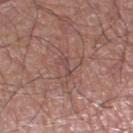Imaged during a routine full-body skin examination; the lesion was not biopsied and no histopathology is available. Cropped from a whole-body photographic skin survey; the tile spans about 15 mm. On the left lower leg. A male patient, aged approximately 60.